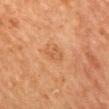Q: Was this lesion biopsied?
A: catalogued during a skin exam; not biopsied
Q: What are the patient's age and sex?
A: female, aged 58–62
Q: What kind of image is this?
A: 15 mm crop, total-body photography
Q: What is the anatomic site?
A: the mid back
Q: What is the lesion's diameter?
A: ~3 mm (longest diameter)
Q: Illumination type?
A: cross-polarized illumination